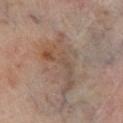Background: On the right lower leg. A male subject, approximately 70 years of age. Measured at roughly 7 mm in maximum diameter. This is a cross-polarized tile. A region of skin cropped from a whole-body photographic capture, roughly 15 mm wide.